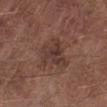The tile uses white-light illumination. Located on the left lower leg. A male patient, aged approximately 55. Cropped from a whole-body photographic skin survey; the tile spans about 15 mm. About 4 mm across.